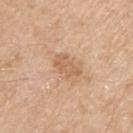Assessment:
Imaged during a routine full-body skin examination; the lesion was not biopsied and no histopathology is available.
Image and clinical context:
A male subject, roughly 70 years of age. The lesion is on the right upper arm. The recorded lesion diameter is about 3.5 mm. A region of skin cropped from a whole-body photographic capture, roughly 15 mm wide.